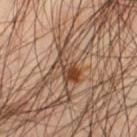Automated image analysis of the tile measured a lesion area of about 6.5 mm², a shape eccentricity near 0.85, and a shape-asymmetry score of about 0.45 (0 = symmetric). The software also gave a lesion-to-skin contrast of about 9.5 (normalized; higher = more distinct). The analysis additionally found a border-irregularity index near 5.5/10, a within-lesion color-variation index near 5.5/10, and radial color variation of about 1.5. It also reported a nevus-likeness score of about 95/100. The subject is a male about 45 years old. The lesion is on the left thigh. The recorded lesion diameter is about 4 mm. Captured under cross-polarized illumination. Cropped from a total-body skin-imaging series; the visible field is about 15 mm.